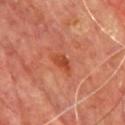Automated tile analysis of the lesion measured border irregularity of about 3 on a 0–10 scale and peripheral color asymmetry of about 1. The software also gave a classifier nevus-likeness of about 65/100 and a lesion-detection confidence of about 100/100.
On the chest.
Cropped from a total-body skin-imaging series; the visible field is about 15 mm.
The recorded lesion diameter is about 3 mm.
A male patient roughly 65 years of age.
Imaged with cross-polarized lighting.